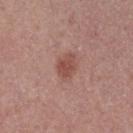Notes:
• biopsy status · catalogued during a skin exam; not biopsied
• imaging modality · 15 mm crop, total-body photography
• patient · female, aged around 40
• site · the right thigh